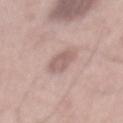Background: Imaged with white-light lighting. A lesion tile, about 15 mm wide, cut from a 3D total-body photograph. Measured at roughly 3 mm in maximum diameter. Located on the mid back. A male subject, aged 53 to 57. The total-body-photography lesion software estimated a border-irregularity rating of about 3/10, a within-lesion color-variation index near 2/10, and peripheral color asymmetry of about 1. It also reported a classifier nevus-likeness of about 0/100 and a lesion-detection confidence of about 100/100.